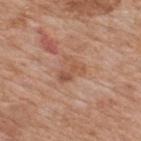Q: Was this lesion biopsied?
A: no biopsy performed (imaged during a skin exam)
Q: What is the imaging modality?
A: 15 mm crop, total-body photography
Q: Where on the body is the lesion?
A: the upper back
Q: What is the lesion's diameter?
A: about 3.5 mm
Q: Who is the patient?
A: male, aged 58–62
Q: Automated lesion metrics?
A: an automated nevus-likeness rating near 0 out of 100 and a detector confidence of about 100 out of 100 that the crop contains a lesion
Q: Illumination type?
A: white-light